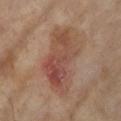Imaged during a routine full-body skin examination; the lesion was not biopsied and no histopathology is available. Imaged with cross-polarized lighting. On the right lower leg. Longest diameter approximately 8 mm. The patient is a female aged approximately 75. A 15 mm close-up tile from a total-body photography series done for melanoma screening.